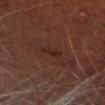Imaged during a routine full-body skin examination; the lesion was not biopsied and no histopathology is available.
Approximately 3 mm at its widest.
A male patient, roughly 65 years of age.
The lesion is on the head or neck.
Cropped from a whole-body photographic skin survey; the tile spans about 15 mm.